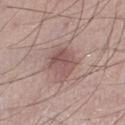<case>
  <biopsy_status>not biopsied; imaged during a skin examination</biopsy_status>
  <patient>
    <sex>male</sex>
    <age_approx>50</age_approx>
  </patient>
  <site>right lower leg</site>
  <image>
    <source>total-body photography crop</source>
    <field_of_view_mm>15</field_of_view_mm>
  </image>
</case>